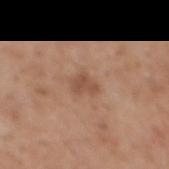image source: total-body-photography crop, ~15 mm field of view
location: the mid back
lesion size: about 2.5 mm
subject: male, aged around 60